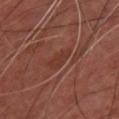Image and clinical context: This is a cross-polarized tile. A male patient aged around 45. The lesion is on the chest. An algorithmic analysis of the crop reported a mean CIELAB color near L≈33 a*≈23 b*≈25, a lesion–skin lightness drop of about 6, and a normalized border contrast of about 5.5. The software also gave border irregularity of about 3 on a 0–10 scale and peripheral color asymmetry of about 0.5. A 15 mm crop from a total-body photograph taken for skin-cancer surveillance.